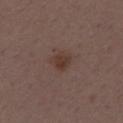notes = catalogued during a skin exam; not biopsied.